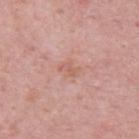biopsy status: total-body-photography surveillance lesion; no biopsy | lesion size: ~2.5 mm (longest diameter) | body site: the back | image: 15 mm crop, total-body photography | subject: male, aged 48–52.